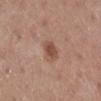Q: Was a biopsy performed?
A: imaged on a skin check; not biopsied
Q: Lesion location?
A: the right lower leg
Q: What is the lesion's diameter?
A: ~2.5 mm (longest diameter)
Q: What is the imaging modality?
A: 15 mm crop, total-body photography
Q: What did automated image analysis measure?
A: a shape eccentricity near 0.7 and two-axis asymmetry of about 0.2; a lesion color around L≈49 a*≈22 b*≈27 in CIELAB and a normalized lesion–skin contrast near 7.5; a border-irregularity rating of about 2/10 and a within-lesion color-variation index near 2/10
Q: Who is the patient?
A: female, about 45 years old
Q: How was the tile lit?
A: white-light illumination